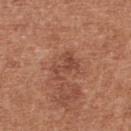Q: Was this lesion biopsied?
A: total-body-photography surveillance lesion; no biopsy
Q: What is the imaging modality?
A: ~15 mm tile from a whole-body skin photo
Q: Lesion location?
A: the upper back
Q: Who is the patient?
A: female, aged approximately 40